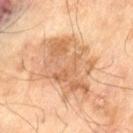Clinical impression: Recorded during total-body skin imaging; not selected for excision or biopsy. Image and clinical context: A 15 mm close-up tile from a total-body photography series done for melanoma screening. The tile uses cross-polarized illumination. Measured at roughly 8.5 mm in maximum diameter. A male subject, aged around 70. From the leg.